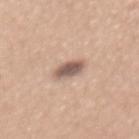Q: Was this lesion biopsied?
A: catalogued during a skin exam; not biopsied
Q: What did automated image analysis measure?
A: border irregularity of about 2 on a 0–10 scale and a within-lesion color-variation index near 3.5/10
Q: What lighting was used for the tile?
A: white-light
Q: How large is the lesion?
A: ≈3 mm
Q: Lesion location?
A: the mid back
Q: Who is the patient?
A: female, aged around 30
Q: How was this image acquired?
A: 15 mm crop, total-body photography